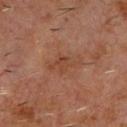Q: Is there a histopathology result?
A: no biopsy performed (imaged during a skin exam)
Q: What is the imaging modality?
A: total-body-photography crop, ~15 mm field of view
Q: Automated lesion metrics?
A: a lesion color around L≈40 a*≈21 b*≈29 in CIELAB, roughly 6 lightness units darker than nearby skin, and a normalized border contrast of about 5.5; a nevus-likeness score of about 0/100 and a detector confidence of about 100 out of 100 that the crop contains a lesion
Q: What lighting was used for the tile?
A: cross-polarized illumination
Q: Where on the body is the lesion?
A: the chest
Q: Patient demographics?
A: male, about 60 years old
Q: Lesion size?
A: ~3.5 mm (longest diameter)